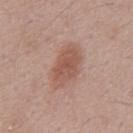workup = no biopsy performed (imaged during a skin exam); lesion diameter = ≈5.5 mm; illumination = white-light illumination; patient = male, roughly 55 years of age; acquisition = ~15 mm tile from a whole-body skin photo.